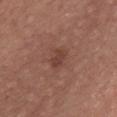notes = catalogued during a skin exam; not biopsied